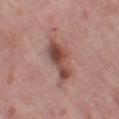Q: Is there a histopathology result?
A: total-body-photography surveillance lesion; no biopsy
Q: Lesion location?
A: the left thigh
Q: What kind of image is this?
A: total-body-photography crop, ~15 mm field of view
Q: How was the tile lit?
A: white-light illumination
Q: Who is the patient?
A: female, aged around 50